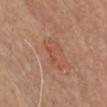follow-up: no biopsy performed (imaged during a skin exam) | imaging modality: 15 mm crop, total-body photography | site: the chest | subject: male, about 60 years old | lesion size: ≈3.5 mm.